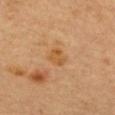Captured during whole-body skin photography for melanoma surveillance; the lesion was not biopsied. A female patient, aged 58–62. Located on the back. A roughly 15 mm field-of-view crop from a total-body skin photograph. Captured under cross-polarized illumination. The recorded lesion diameter is about 2.5 mm.